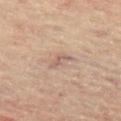Q: Was this lesion biopsied?
A: no biopsy performed (imaged during a skin exam)
Q: What is the anatomic site?
A: the left thigh
Q: Automated lesion metrics?
A: an average lesion color of about L≈52 a*≈16 b*≈23 (CIELAB), roughly 7 lightness units darker than nearby skin, and a lesion-to-skin contrast of about 5.5 (normalized; higher = more distinct); a border-irregularity rating of about 3.5/10, a color-variation rating of about 1/10, and a peripheral color-asymmetry measure near 0; a classifier nevus-likeness of about 0/100 and a lesion-detection confidence of about 75/100
Q: How large is the lesion?
A: ~3 mm (longest diameter)
Q: Patient demographics?
A: male, about 60 years old
Q: How was this image acquired?
A: 15 mm crop, total-body photography
Q: Illumination type?
A: cross-polarized illumination